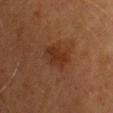The lesion was tiled from a total-body skin photograph and was not biopsied.